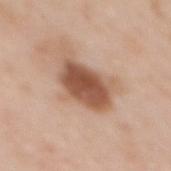Q: Was this lesion biopsied?
A: imaged on a skin check; not biopsied
Q: What are the patient's age and sex?
A: female, in their 50s
Q: Where on the body is the lesion?
A: the mid back
Q: How was this image acquired?
A: total-body-photography crop, ~15 mm field of view